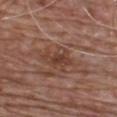Q: Was this lesion biopsied?
A: imaged on a skin check; not biopsied
Q: How was this image acquired?
A: total-body-photography crop, ~15 mm field of view
Q: What is the lesion's diameter?
A: about 4 mm
Q: Lesion location?
A: the chest
Q: What are the patient's age and sex?
A: male, about 65 years old
Q: Automated lesion metrics?
A: a border-irregularity index near 4.5/10 and radial color variation of about 1
Q: What lighting was used for the tile?
A: white-light